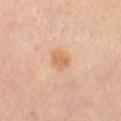The lesion was tiled from a total-body skin photograph and was not biopsied.
On the front of the torso.
A region of skin cropped from a whole-body photographic capture, roughly 15 mm wide.
A female patient, aged approximately 40.
Approximately 2.5 mm at its widest.